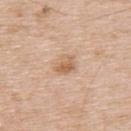Findings:
* workup · catalogued during a skin exam; not biopsied
* subject · male, about 65 years old
* tile lighting · white-light illumination
* automated lesion analysis · an area of roughly 5 mm² and an outline eccentricity of about 0.6 (0 = round, 1 = elongated); roughly 10 lightness units darker than nearby skin and a normalized border contrast of about 6.5; a classifier nevus-likeness of about 35/100 and a lesion-detection confidence of about 100/100
* imaging modality · ~15 mm crop, total-body skin-cancer survey
* lesion size · ~3 mm (longest diameter)
* site · the upper back